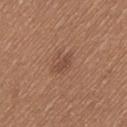{
  "biopsy_status": "not biopsied; imaged during a skin examination",
  "image": {
    "source": "total-body photography crop",
    "field_of_view_mm": 15
  },
  "site": "left thigh",
  "patient": {
    "sex": "male",
    "age_approx": 65
  }
}